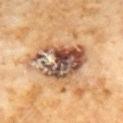No biopsy was performed on this lesion — it was imaged during a full skin examination and was not determined to be concerning. A male subject aged 58–62. The lesion-visualizer software estimated an average lesion color of about L≈52 a*≈19 b*≈30 (CIELAB) and a lesion–skin lightness drop of about 17. The analysis additionally found a classifier nevus-likeness of about 0/100. A close-up tile cropped from a whole-body skin photograph, about 15 mm across. From the chest. This is a cross-polarized tile. Measured at roughly 7.5 mm in maximum diameter.